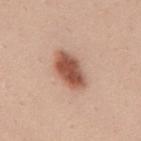Q: Is there a histopathology result?
A: total-body-photography surveillance lesion; no biopsy
Q: What is the lesion's diameter?
A: about 5 mm
Q: How was this image acquired?
A: ~15 mm tile from a whole-body skin photo
Q: What is the anatomic site?
A: the mid back
Q: Automated lesion metrics?
A: a border-irregularity rating of about 1.5/10 and peripheral color asymmetry of about 1.5; an automated nevus-likeness rating near 100 out of 100
Q: What are the patient's age and sex?
A: female, aged around 25